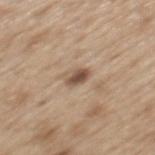notes — no biopsy performed (imaged during a skin exam) | acquisition — total-body-photography crop, ~15 mm field of view | size — ≈2.5 mm | subject — male, aged 68–72 | automated metrics — an area of roughly 3.5 mm², an eccentricity of roughly 0.7, and a shape-asymmetry score of about 0.25 (0 = symmetric); a lesion color around L≈51 a*≈16 b*≈28 in CIELAB, roughly 13 lightness units darker than nearby skin, and a normalized border contrast of about 9.5; a border-irregularity index near 2.5/10 and a peripheral color-asymmetry measure near 1.5 | tile lighting — white-light illumination | body site — the mid back.